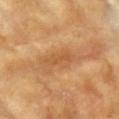Measured at roughly 4 mm in maximum diameter.
A female subject aged 73–77.
A region of skin cropped from a whole-body photographic capture, roughly 15 mm wide.
From the arm.
Captured under cross-polarized illumination.